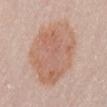| field | value |
|---|---|
| workup | no biopsy performed (imaged during a skin exam) |
| site | the lower back |
| tile lighting | white-light |
| acquisition | total-body-photography crop, ~15 mm field of view |
| automated lesion analysis | a lesion area of about 42 mm², an eccentricity of roughly 0.6, and two-axis asymmetry of about 0.15 |
| patient | female, aged 58 to 62 |